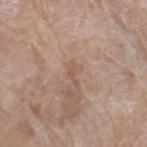A 15 mm crop from a total-body photograph taken for skin-cancer surveillance. The lesion is on the right thigh. Automated image analysis of the tile measured a within-lesion color-variation index near 0/10 and radial color variation of about 0. The software also gave a classifier nevus-likeness of about 0/100 and lesion-presence confidence of about 100/100. A female subject, in their mid-70s.